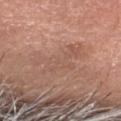biopsy status = catalogued during a skin exam; not biopsied
site = the head or neck
acquisition = ~15 mm tile from a whole-body skin photo
tile lighting = white-light illumination
subject = male, about 55 years old
image-analysis metrics = a border-irregularity index near 9.5/10 and a color-variation rating of about 2.5/10
lesion diameter = about 6.5 mm